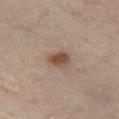Assessment:
Imaged during a routine full-body skin examination; the lesion was not biopsied and no histopathology is available.